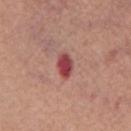<case>
  <biopsy_status>not biopsied; imaged during a skin examination</biopsy_status>
  <lesion_size>
    <long_diameter_mm_approx>3.5</long_diameter_mm_approx>
  </lesion_size>
  <image>
    <source>total-body photography crop</source>
    <field_of_view_mm>15</field_of_view_mm>
  </image>
  <lighting>white-light</lighting>
  <site>chest</site>
  <patient>
    <sex>male</sex>
    <age_approx>65</age_approx>
  </patient>
</case>